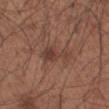The lesion was photographed on a routine skin check and not biopsied; there is no pathology result. This image is a 15 mm lesion crop taken from a total-body photograph. The tile uses white-light illumination. About 4.5 mm across. An algorithmic analysis of the crop reported an area of roughly 7 mm² and a shape eccentricity near 0.85. The analysis additionally found a lesion color around L≈40 a*≈20 b*≈25 in CIELAB, a lesion–skin lightness drop of about 7, and a lesion-to-skin contrast of about 6.5 (normalized; higher = more distinct). The software also gave a classifier nevus-likeness of about 35/100 and a lesion-detection confidence of about 100/100. The lesion is on the left forearm. A male subject, roughly 55 years of age.